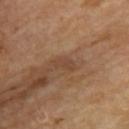Notes:
• notes: catalogued during a skin exam; not biopsied
• image source: ~15 mm crop, total-body skin-cancer survey
• body site: the upper back
• automated lesion analysis: roughly 6 lightness units darker than nearby skin and a normalized border contrast of about 5; border irregularity of about 3 on a 0–10 scale, a color-variation rating of about 0.5/10, and a peripheral color-asymmetry measure near 0
• size: ≈2.5 mm
• patient: female, aged approximately 65